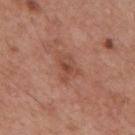{"biopsy_status": "not biopsied; imaged during a skin examination", "automated_metrics": {"border_irregularity_0_10": 3.5, "color_variation_0_10": 4.0, "peripheral_color_asymmetry": 1.0, "nevus_likeness_0_100": 0, "lesion_detection_confidence_0_100": 100}, "patient": {"sex": "male", "age_approx": 55}, "image": {"source": "total-body photography crop", "field_of_view_mm": 15}, "site": "mid back", "lighting": "white-light", "lesion_size": {"long_diameter_mm_approx": 3.5}}